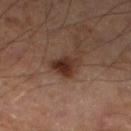Assessment:
The lesion was tiled from a total-body skin photograph and was not biopsied.
Clinical summary:
Located on the left lower leg. The tile uses cross-polarized illumination. A male subject, roughly 55 years of age. A 15 mm crop from a total-body photograph taken for skin-cancer surveillance. The recorded lesion diameter is about 3.5 mm. An algorithmic analysis of the crop reported a footprint of about 6.5 mm², an outline eccentricity of about 0.7 (0 = round, 1 = elongated), and a shape-asymmetry score of about 0.3 (0 = symmetric). It also reported a lesion color around L≈29 a*≈18 b*≈23 in CIELAB, about 11 CIELAB-L* units darker than the surrounding skin, and a normalized border contrast of about 11. It also reported a border-irregularity index near 2.5/10, internal color variation of about 5 on a 0–10 scale, and peripheral color asymmetry of about 2.